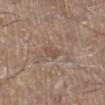{
  "biopsy_status": "not biopsied; imaged during a skin examination"
}